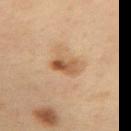Captured during whole-body skin photography for melanoma surveillance; the lesion was not biopsied.
Longest diameter approximately 3 mm.
A lesion tile, about 15 mm wide, cut from a 3D total-body photograph.
Captured under cross-polarized illumination.
The lesion is on the left forearm.
A male patient, roughly 60 years of age.
The lesion-visualizer software estimated a footprint of about 5 mm², a shape eccentricity near 0.75, and two-axis asymmetry of about 0.2. The analysis additionally found a mean CIELAB color near L≈54 a*≈20 b*≈35, roughly 12 lightness units darker than nearby skin, and a normalized border contrast of about 8. The analysis additionally found a border-irregularity index near 2.5/10, internal color variation of about 8.5 on a 0–10 scale, and a peripheral color-asymmetry measure near 3.5.